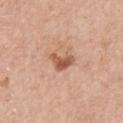biopsy_status: not biopsied; imaged during a skin examination
site: left arm
lesion_size:
  long_diameter_mm_approx: 3.5
lighting: white-light
image:
  source: total-body photography crop
  field_of_view_mm: 15
patient:
  sex: male
  age_approx: 80